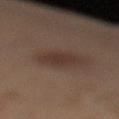Captured during whole-body skin photography for melanoma surveillance; the lesion was not biopsied. On the left lower leg. Measured at roughly 3.5 mm in maximum diameter. A female patient, in their 40s. Cropped from a total-body skin-imaging series; the visible field is about 15 mm.